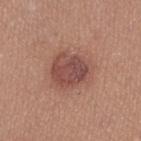{
  "biopsy_status": "not biopsied; imaged during a skin examination",
  "patient": {
    "sex": "female",
    "age_approx": 35
  },
  "site": "left lower leg",
  "image": {
    "source": "total-body photography crop",
    "field_of_view_mm": 15
  }
}